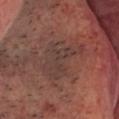biopsy_status: not biopsied; imaged during a skin examination
patient:
  age_approx: 55
site: head or neck
image:
  source: total-body photography crop
  field_of_view_mm: 15
lighting: cross-polarized